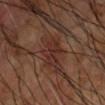Recorded during total-body skin imaging; not selected for excision or biopsy. Cropped from a total-body skin-imaging series; the visible field is about 15 mm. Measured at roughly 4 mm in maximum diameter. From the right forearm. A male subject approximately 65 years of age. This is a cross-polarized tile.